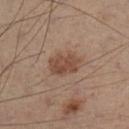| field | value |
|---|---|
| lesion size | ≈4 mm |
| image source | ~15 mm tile from a whole-body skin photo |
| lighting | cross-polarized |
| location | the left leg |
| automated metrics | a border-irregularity rating of about 2/10 and a color-variation rating of about 2.5/10; a detector confidence of about 100 out of 100 that the crop contains a lesion |
| subject | male, approximately 50 years of age |